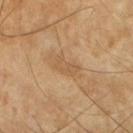Clinical impression:
The lesion was tiled from a total-body skin photograph and was not biopsied.
Acquisition and patient details:
The lesion is on the chest. A male subject, in their 60s. A 15 mm close-up tile from a total-body photography series done for melanoma screening.